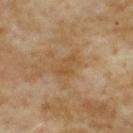| field | value |
|---|---|
| follow-up | catalogued during a skin exam; not biopsied |
| illumination | cross-polarized illumination |
| lesion size | ≈3.5 mm |
| TBP lesion metrics | a within-lesion color-variation index near 1.5/10 and peripheral color asymmetry of about 0.5 |
| imaging modality | ~15 mm tile from a whole-body skin photo |
| location | the back |
| patient | female, aged 58–62 |